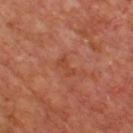notes = total-body-photography surveillance lesion; no biopsy
lighting = cross-polarized
location = the chest
TBP lesion metrics = two-axis asymmetry of about 0.35; a lesion color around L≈43 a*≈28 b*≈32 in CIELAB and roughly 6 lightness units darker than nearby skin; border irregularity of about 4 on a 0–10 scale, internal color variation of about 0 on a 0–10 scale, and peripheral color asymmetry of about 0
imaging modality = ~15 mm tile from a whole-body skin photo
subject = male, aged 63 to 67